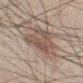The lesion was photographed on a routine skin check and not biopsied; there is no pathology result.